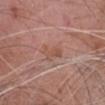Part of a total-body skin-imaging series; this lesion was reviewed on a skin check and was not flagged for biopsy. The tile uses white-light illumination. On the head or neck. Approximately 3 mm at its widest. The total-body-photography lesion software estimated an average lesion color of about L≈52 a*≈22 b*≈27 (CIELAB), about 7 CIELAB-L* units darker than the surrounding skin, and a lesion-to-skin contrast of about 5.5 (normalized; higher = more distinct). The software also gave a nevus-likeness score of about 0/100 and lesion-presence confidence of about 100/100. A male patient aged around 75. A 15 mm close-up extracted from a 3D total-body photography capture.